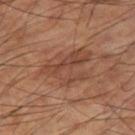Assessment:
Captured during whole-body skin photography for melanoma surveillance; the lesion was not biopsied.
Clinical summary:
The patient is a male about 60 years old. A 15 mm close-up tile from a total-body photography series done for melanoma screening. From the right thigh. Imaged with cross-polarized lighting.